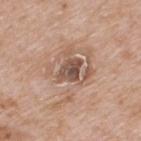Clinical impression: The lesion was photographed on a routine skin check and not biopsied; there is no pathology result. Clinical summary: The lesion-visualizer software estimated an area of roughly 5 mm² and two-axis asymmetry of about 0.2. And it measured a mean CIELAB color near L≈50 a*≈18 b*≈25 and roughly 12 lightness units darker than nearby skin. And it measured a peripheral color-asymmetry measure near 2. Cropped from a total-body skin-imaging series; the visible field is about 15 mm. Located on the upper back. Longest diameter approximately 3 mm. A male subject roughly 65 years of age.